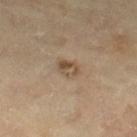biopsy status = no biopsy performed (imaged during a skin exam) | site = the right thigh | imaging modality = ~15 mm tile from a whole-body skin photo | subject = male, aged 63–67.